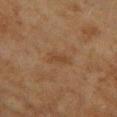Impression: Captured during whole-body skin photography for melanoma surveillance; the lesion was not biopsied.